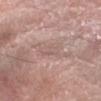Captured during whole-body skin photography for melanoma surveillance; the lesion was not biopsied.
Automated image analysis of the tile measured a lesion area of about 4.5 mm² and an outline eccentricity of about 0.75 (0 = round, 1 = elongated). It also reported a detector confidence of about 20 out of 100 that the crop contains a lesion.
The recorded lesion diameter is about 3 mm.
A region of skin cropped from a whole-body photographic capture, roughly 15 mm wide.
A male patient, about 70 years old.
On the left forearm.
The tile uses white-light illumination.